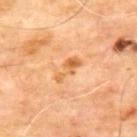Context:
The tile uses cross-polarized illumination. The patient is a male roughly 65 years of age. A 15 mm close-up extracted from a 3D total-body photography capture. Longest diameter approximately 3.5 mm. The lesion is on the back.